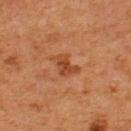notes: no biopsy performed (imaged during a skin exam)
image-analysis metrics: a footprint of about 5.5 mm², a shape eccentricity near 0.8, and a shape-asymmetry score of about 0.4 (0 = symmetric); a border-irregularity index near 4.5/10, a within-lesion color-variation index near 2.5/10, and a peripheral color-asymmetry measure near 1; a nevus-likeness score of about 20/100 and a detector confidence of about 100 out of 100 that the crop contains a lesion
lesion size: ~3.5 mm (longest diameter)
body site: the upper back
patient: male, aged approximately 65
lighting: cross-polarized
acquisition: ~15 mm tile from a whole-body skin photo